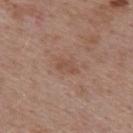Q: Was a biopsy performed?
A: imaged on a skin check; not biopsied
Q: What is the imaging modality?
A: ~15 mm crop, total-body skin-cancer survey
Q: What are the patient's age and sex?
A: female, about 40 years old
Q: What is the anatomic site?
A: the back
Q: Illumination type?
A: white-light